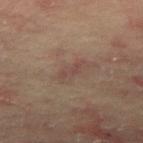Clinical impression:
Captured during whole-body skin photography for melanoma surveillance; the lesion was not biopsied.
Background:
Cropped from a whole-body photographic skin survey; the tile spans about 15 mm. The lesion is located on the right thigh. A male patient aged around 65.